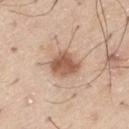Impression: No biopsy was performed on this lesion — it was imaged during a full skin examination and was not determined to be concerning. Context: Cropped from a total-body skin-imaging series; the visible field is about 15 mm. On the left thigh. A male patient approximately 60 years of age. The tile uses white-light illumination.